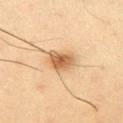biopsy status: catalogued during a skin exam; not biopsied | lesion diameter: about 3.5 mm | subject: male, in their mid-30s | location: the left upper arm | tile lighting: cross-polarized | image: ~15 mm crop, total-body skin-cancer survey.